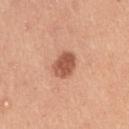{
  "biopsy_status": "not biopsied; imaged during a skin examination",
  "patient": {
    "sex": "female",
    "age_approx": 45
  },
  "image": {
    "source": "total-body photography crop",
    "field_of_view_mm": 15
  },
  "site": "right upper arm",
  "automated_metrics": {
    "border_irregularity_0_10": 1.5,
    "color_variation_0_10": 2.5,
    "peripheral_color_asymmetry": 1.0,
    "nevus_likeness_0_100": 95
  },
  "lesion_size": {
    "long_diameter_mm_approx": 3.0
  }
}